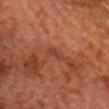Part of a total-body skin-imaging series; this lesion was reviewed on a skin check and was not flagged for biopsy.
A 15 mm close-up extracted from a 3D total-body photography capture.
The lesion is on the chest.
A male subject, roughly 80 years of age.
This is a cross-polarized tile.
Approximately 2.5 mm at its widest.